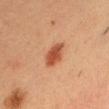Captured during whole-body skin photography for melanoma surveillance; the lesion was not biopsied.
From the head or neck.
A male patient aged around 35.
The lesion-visualizer software estimated a lesion color around L≈46 a*≈27 b*≈34 in CIELAB, a lesion–skin lightness drop of about 12, and a lesion-to-skin contrast of about 9.5 (normalized; higher = more distinct). It also reported a nevus-likeness score of about 100/100 and lesion-presence confidence of about 100/100.
Imaged with cross-polarized lighting.
Approximately 4 mm at its widest.
A roughly 15 mm field-of-view crop from a total-body skin photograph.